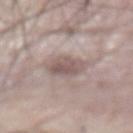<lesion>
  <biopsy_status>not biopsied; imaged during a skin examination</biopsy_status>
  <lesion_size>
    <long_diameter_mm_approx>3.5</long_diameter_mm_approx>
  </lesion_size>
  <automated_metrics>
    <cielab_L>54</cielab_L>
    <cielab_a>15</cielab_a>
    <cielab_b>19</cielab_b>
    <vs_skin_contrast_norm>7.0</vs_skin_contrast_norm>
    <nevus_likeness_0_100>10</nevus_likeness_0_100>
    <lesion_detection_confidence_0_100>65</lesion_detection_confidence_0_100>
  </automated_metrics>
  <patient>
    <sex>male</sex>
    <age_approx>65</age_approx>
  </patient>
  <lighting>white-light</lighting>
  <site>abdomen</site>
  <image>
    <source>total-body photography crop</source>
    <field_of_view_mm>15</field_of_view_mm>
  </image>
</lesion>